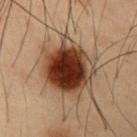Imaged during a routine full-body skin examination; the lesion was not biopsied and no histopathology is available. A male subject, in their mid- to late 50s. The lesion is located on the abdomen. Cropped from a total-body skin-imaging series; the visible field is about 15 mm.